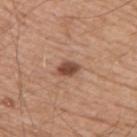{"biopsy_status": "not biopsied; imaged during a skin examination", "patient": {"sex": "male", "age_approx": 55}, "image": {"source": "total-body photography crop", "field_of_view_mm": 15}, "site": "back", "lesion_size": {"long_diameter_mm_approx": 2.5}, "automated_metrics": {"area_mm2_approx": 4.0, "eccentricity": 0.7, "shape_asymmetry": 0.25, "cielab_L": 47, "cielab_a": 22, "cielab_b": 29, "vs_skin_darker_L": 14.0, "vs_skin_contrast_norm": 10.0, "border_irregularity_0_10": 2.0, "color_variation_0_10": 2.5, "peripheral_color_asymmetry": 1.0, "nevus_likeness_0_100": 95}, "lighting": "white-light"}